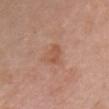Approximately 2.5 mm at its widest.
A female patient, aged approximately 50.
An algorithmic analysis of the crop reported an area of roughly 4 mm² and a shape eccentricity near 0.8. The software also gave about 7 CIELAB-L* units darker than the surrounding skin and a lesion-to-skin contrast of about 6 (normalized; higher = more distinct). The analysis additionally found a lesion-detection confidence of about 100/100.
The tile uses white-light illumination.
From the chest.
A 15 mm close-up extracted from a 3D total-body photography capture.